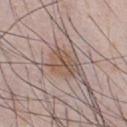Recorded during total-body skin imaging; not selected for excision or biopsy. A 15 mm crop from a total-body photograph taken for skin-cancer surveillance. Captured under white-light illumination. The total-body-photography lesion software estimated a footprint of about 7 mm², a shape eccentricity near 0.7, and two-axis asymmetry of about 0.35. The recorded lesion diameter is about 3.5 mm. On the front of the torso. A male subject, in their mid-30s.